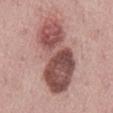Imaged during a routine full-body skin examination; the lesion was not biopsied and no histopathology is available. A male patient, aged around 45. Captured under white-light illumination. Automated image analysis of the tile measured a mean CIELAB color near L≈50 a*≈23 b*≈22, a lesion–skin lightness drop of about 17, and a normalized lesion–skin contrast near 11. It also reported a border-irregularity index near 4/10, a color-variation rating of about 8.5/10, and peripheral color asymmetry of about 3.5. And it measured a classifier nevus-likeness of about 5/100 and lesion-presence confidence of about 100/100. The lesion is located on the mid back. A lesion tile, about 15 mm wide, cut from a 3D total-body photograph. The lesion's longest dimension is about 12 mm.